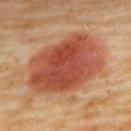Findings:
• notes — catalogued during a skin exam; not biopsied